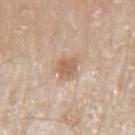Part of a total-body skin-imaging series; this lesion was reviewed on a skin check and was not flagged for biopsy. A region of skin cropped from a whole-body photographic capture, roughly 15 mm wide. Approximately 3 mm at its widest. An algorithmic analysis of the crop reported an area of roughly 5 mm², an outline eccentricity of about 0.7 (0 = round, 1 = elongated), and a shape-asymmetry score of about 0.25 (0 = symmetric). The software also gave about 10 CIELAB-L* units darker than the surrounding skin and a normalized border contrast of about 7. And it measured a border-irregularity index near 3/10, a within-lesion color-variation index near 2.5/10, and peripheral color asymmetry of about 1. It also reported an automated nevus-likeness rating near 20 out of 100 and lesion-presence confidence of about 100/100. Imaged with white-light lighting. From the arm. A male patient, aged 78 to 82.